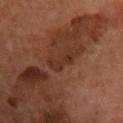Notes:
– notes · no biopsy performed (imaged during a skin exam)
– TBP lesion metrics · a lesion area of about 4.5 mm² and an eccentricity of roughly 0.9; a nevus-likeness score of about 0/100
– size · ≈3.5 mm
– acquisition · 15 mm crop, total-body photography
– illumination · cross-polarized illumination
– body site · the front of the torso
– patient · male, aged approximately 65